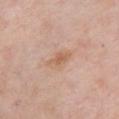biopsy_status: not biopsied; imaged during a skin examination
patient:
  sex: female
  age_approx: 60
site: front of the torso
automated_metrics:
  area_mm2_approx: 4.5
  eccentricity: 0.85
image:
  source: total-body photography crop
  field_of_view_mm: 15
lesion_size:
  long_diameter_mm_approx: 3.5